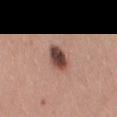Q: Is there a histopathology result?
A: no biopsy performed (imaged during a skin exam)
Q: Lesion location?
A: the left thigh
Q: What lighting was used for the tile?
A: white-light illumination
Q: Patient demographics?
A: female, aged 63 to 67
Q: What is the lesion's diameter?
A: about 3.5 mm
Q: What kind of image is this?
A: total-body-photography crop, ~15 mm field of view
Q: What did automated image analysis measure?
A: a classifier nevus-likeness of about 100/100